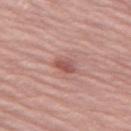Q: Is there a histopathology result?
A: no biopsy performed (imaged during a skin exam)
Q: Illumination type?
A: white-light illumination
Q: Patient demographics?
A: female, approximately 70 years of age
Q: Where on the body is the lesion?
A: the left thigh
Q: What is the imaging modality?
A: total-body-photography crop, ~15 mm field of view
Q: What did automated image analysis measure?
A: a border-irregularity index near 3.5/10, a color-variation rating of about 2.5/10, and a peripheral color-asymmetry measure near 0.5